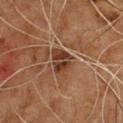biopsy_status: not biopsied; imaged during a skin examination
automated_metrics:
  area_mm2_approx: 6.5
  shape_asymmetry: 0.3
image:
  source: total-body photography crop
  field_of_view_mm: 15
lighting: cross-polarized
patient:
  sex: male
  age_approx: 60
site: front of the torso